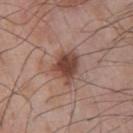Notes:
* notes: catalogued during a skin exam; not biopsied
* acquisition: 15 mm crop, total-body photography
* tile lighting: white-light illumination
* subject: male, in their mid-60s
* size: ≈3.5 mm
* location: the chest
* TBP lesion metrics: an average lesion color of about L≈44 a*≈21 b*≈25 (CIELAB) and a normalized border contrast of about 9.5; a border-irregularity index near 3/10, a within-lesion color-variation index near 4.5/10, and radial color variation of about 1.5